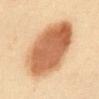{"patient": {"sex": "female", "age_approx": 40}, "site": "abdomen", "image": {"source": "total-body photography crop", "field_of_view_mm": 15}}